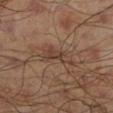notes: no biopsy performed (imaged during a skin exam); image: 15 mm crop, total-body photography; size: ~4.5 mm (longest diameter); illumination: cross-polarized illumination; site: the left lower leg; subject: male, in their mid-40s; automated metrics: an area of roughly 6.5 mm², an eccentricity of roughly 0.9, and a shape-asymmetry score of about 0.5 (0 = symmetric).